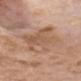Clinical impression:
No biopsy was performed on this lesion — it was imaged during a full skin examination and was not determined to be concerning.
Background:
Measured at roughly 8 mm in maximum diameter. Captured under white-light illumination. This image is a 15 mm lesion crop taken from a total-body photograph. The patient is a male aged 78 to 82. The lesion is located on the head or neck.